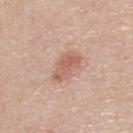{
  "biopsy_status": "not biopsied; imaged during a skin examination",
  "lighting": "white-light",
  "lesion_size": {
    "long_diameter_mm_approx": 4.0
  },
  "patient": {
    "sex": "female",
    "age_approx": 55
  },
  "automated_metrics": {
    "vs_skin_darker_L": 10.0,
    "vs_skin_contrast_norm": 6.5,
    "nevus_likeness_0_100": 45,
    "lesion_detection_confidence_0_100": 100
  },
  "site": "upper back",
  "image": {
    "source": "total-body photography crop",
    "field_of_view_mm": 15
  }
}